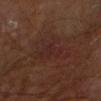biopsy_status: not biopsied; imaged during a skin examination
image:
  source: total-body photography crop
  field_of_view_mm: 15
site: right forearm
patient:
  sex: male
  age_approx: 65
lighting: cross-polarized